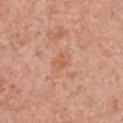{"biopsy_status": "not biopsied; imaged during a skin examination", "image": {"source": "total-body photography crop", "field_of_view_mm": 15}, "patient": {"sex": "female", "age_approx": 60}, "lighting": "white-light", "site": "front of the torso"}